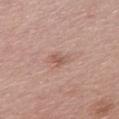Clinical impression: The lesion was photographed on a routine skin check and not biopsied; there is no pathology result. Clinical summary: A female patient aged 63–67. Longest diameter approximately 2.5 mm. On the chest. The total-body-photography lesion software estimated a lesion area of about 2.5 mm² and a symmetry-axis asymmetry near 0.4. The analysis additionally found a border-irregularity index near 4/10 and radial color variation of about 0. And it measured a nevus-likeness score of about 0/100 and lesion-presence confidence of about 100/100. A 15 mm crop from a total-body photograph taken for skin-cancer surveillance. Imaged with white-light lighting.